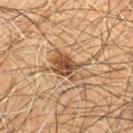Clinical impression: The lesion was photographed on a routine skin check and not biopsied; there is no pathology result. Acquisition and patient details: On the chest. The recorded lesion diameter is about 4.5 mm. Captured under cross-polarized illumination. A male patient approximately 60 years of age. A 15 mm close-up tile from a total-body photography series done for melanoma screening.